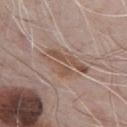| field | value |
|---|---|
| follow-up | total-body-photography surveillance lesion; no biopsy |
| body site | the front of the torso |
| image source | ~15 mm tile from a whole-body skin photo |
| lesion diameter | ≈6 mm |
| illumination | white-light illumination |
| image-analysis metrics | an area of roughly 12 mm², an eccentricity of roughly 0.9, and a symmetry-axis asymmetry near 0.4; a nevus-likeness score of about 0/100 and a detector confidence of about 60 out of 100 that the crop contains a lesion |
| subject | male, aged approximately 70 |